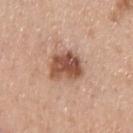<case>
  <biopsy_status>not biopsied; imaged during a skin examination</biopsy_status>
</case>